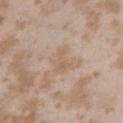Case summary:
- follow-up: catalogued during a skin exam; not biopsied